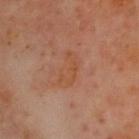Part of a total-body skin-imaging series; this lesion was reviewed on a skin check and was not flagged for biopsy. A lesion tile, about 15 mm wide, cut from a 3D total-body photograph. Captured under cross-polarized illumination. The subject is a male aged 63–67. The lesion is on the head or neck.